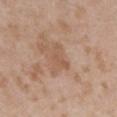<case>
<patient>
  <sex>female</sex>
  <age_approx>30</age_approx>
</patient>
<site>mid back</site>
<image>
  <source>total-body photography crop</source>
  <field_of_view_mm>15</field_of_view_mm>
</image>
</case>